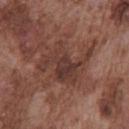Assessment: Captured during whole-body skin photography for melanoma surveillance; the lesion was not biopsied. Background: This is a white-light tile. The total-body-photography lesion software estimated a lesion area of about 17 mm², an outline eccentricity of about 0.6 (0 = round, 1 = elongated), and two-axis asymmetry of about 0.45. And it measured a mean CIELAB color near L≈37 a*≈20 b*≈23, roughly 8 lightness units darker than nearby skin, and a lesion-to-skin contrast of about 7 (normalized; higher = more distinct). It also reported border irregularity of about 8.5 on a 0–10 scale, internal color variation of about 4.5 on a 0–10 scale, and a peripheral color-asymmetry measure near 1. This image is a 15 mm lesion crop taken from a total-body photograph. A male patient aged 73 to 77. The lesion is located on the front of the torso.